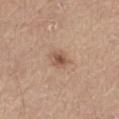Recorded during total-body skin imaging; not selected for excision or biopsy.
A region of skin cropped from a whole-body photographic capture, roughly 15 mm wide.
The patient is a male in their mid-60s.
Located on the abdomen.